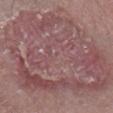Impression: Imaged during a routine full-body skin examination; the lesion was not biopsied and no histopathology is available. Acquisition and patient details: The lesion is located on the left lower leg. Longest diameter approximately 14 mm. The patient is a male aged approximately 80. A 15 mm close-up extracted from a 3D total-body photography capture. The tile uses white-light illumination.